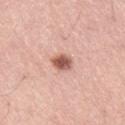Recorded during total-body skin imaging; not selected for excision or biopsy.
The lesion is located on the right thigh.
A 15 mm close-up extracted from a 3D total-body photography capture.
The total-body-photography lesion software estimated a lesion area of about 4.5 mm², a shape eccentricity near 0.65, and a shape-asymmetry score of about 0.15 (0 = symmetric). The analysis additionally found border irregularity of about 1.5 on a 0–10 scale and a within-lesion color-variation index near 4/10. And it measured a classifier nevus-likeness of about 100/100 and a lesion-detection confidence of about 100/100.
Longest diameter approximately 3 mm.
The patient is a male approximately 50 years of age.
This is a white-light tile.